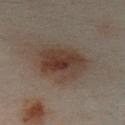Part of a total-body skin-imaging series; this lesion was reviewed on a skin check and was not flagged for biopsy.
Automated tile analysis of the lesion measured a nevus-likeness score of about 90/100 and lesion-presence confidence of about 100/100.
A 15 mm crop from a total-body photograph taken for skin-cancer surveillance.
The lesion is located on the left lower leg.
This is a cross-polarized tile.
About 6 mm across.
A male patient approximately 50 years of age.